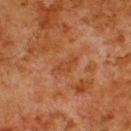* follow-up — catalogued during a skin exam; not biopsied
* acquisition — 15 mm crop, total-body photography
* patient — male, aged 78–82
* location — the back